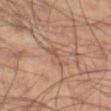Part of a total-body skin-imaging series; this lesion was reviewed on a skin check and was not flagged for biopsy. A region of skin cropped from a whole-body photographic capture, roughly 15 mm wide. Captured under cross-polarized illumination. From the left thigh. The patient is a male in their 60s.